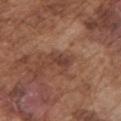On the left upper arm.
A male subject in their mid- to late 70s.
Approximately 3 mm at its widest.
A 15 mm crop from a total-body photograph taken for skin-cancer surveillance.
Captured under white-light illumination.